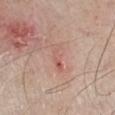Clinical impression: No biopsy was performed on this lesion — it was imaged during a full skin examination and was not determined to be concerning. Image and clinical context: The lesion-visualizer software estimated a border-irregularity rating of about 5/10, a within-lesion color-variation index near 1.5/10, and peripheral color asymmetry of about 0.5. The software also gave a nevus-likeness score of about 0/100 and lesion-presence confidence of about 100/100. The lesion is located on the chest. Measured at roughly 3.5 mm in maximum diameter. This image is a 15 mm lesion crop taken from a total-body photograph. The patient is a male roughly 70 years of age.